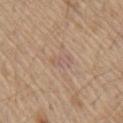workup=no biopsy performed (imaged during a skin exam)
size=≈2.5 mm
acquisition=~15 mm tile from a whole-body skin photo
subject=male, aged 68 to 72
image-analysis metrics=a lesion color around L≈58 a*≈17 b*≈25 in CIELAB, a lesion–skin lightness drop of about 6, and a normalized lesion–skin contrast near 4.5; border irregularity of about 3.5 on a 0–10 scale and radial color variation of about 0
tile lighting=white-light illumination
location=the chest